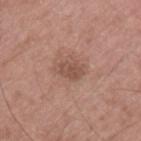Background:
A 15 mm crop from a total-body photograph taken for skin-cancer surveillance. The lesion is located on the upper back. Longest diameter approximately 3.5 mm. This is a white-light tile. The patient is a male aged 58–62.